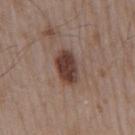No biopsy was performed on this lesion — it was imaged during a full skin examination and was not determined to be concerning. Approximately 4.5 mm at its widest. The lesion-visualizer software estimated two-axis asymmetry of about 0.1. The lesion is located on the right upper arm. Imaged with white-light lighting. The subject is a male roughly 55 years of age. A 15 mm close-up extracted from a 3D total-body photography capture.